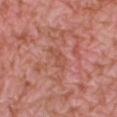| key | value |
|---|---|
| workup | no biopsy performed (imaged during a skin exam) |
| automated lesion analysis | border irregularity of about 4.5 on a 0–10 scale, a within-lesion color-variation index near 1/10, and radial color variation of about 0.5; an automated nevus-likeness rating near 0 out of 100 |
| size | about 3.5 mm |
| location | the right lower leg |
| imaging modality | total-body-photography crop, ~15 mm field of view |
| lighting | white-light |
| patient | female, aged 38–42 |